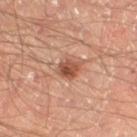A close-up tile cropped from a whole-body skin photograph, about 15 mm across. The lesion-visualizer software estimated a lesion area of about 4.5 mm² and a symmetry-axis asymmetry near 0.2. The analysis additionally found a classifier nevus-likeness of about 90/100 and a detector confidence of about 100 out of 100 that the crop contains a lesion. A male subject aged around 65. Imaged with cross-polarized lighting. Located on the right thigh.